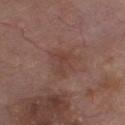The lesion's longest dimension is about 3 mm.
Automated image analysis of the tile measured a footprint of about 5 mm², a shape eccentricity near 0.7, and a symmetry-axis asymmetry near 0.45.
Imaged with white-light lighting.
From the front of the torso.
The patient is a male aged around 80.
A 15 mm crop from a total-body photograph taken for skin-cancer surveillance.